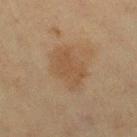Q: Was this lesion biopsied?
A: no biopsy performed (imaged during a skin exam)
Q: What kind of image is this?
A: total-body-photography crop, ~15 mm field of view
Q: Patient demographics?
A: female, in their 50s
Q: Where on the body is the lesion?
A: the right thigh